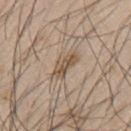Clinical impression:
Imaged during a routine full-body skin examination; the lesion was not biopsied and no histopathology is available.
Image and clinical context:
Imaged with white-light lighting. An algorithmic analysis of the crop reported a footprint of about 5 mm² and an outline eccentricity of about 0.9 (0 = round, 1 = elongated). It also reported a border-irregularity rating of about 4/10, a within-lesion color-variation index near 2/10, and peripheral color asymmetry of about 0.5. Located on the back. The recorded lesion diameter is about 3.5 mm. A 15 mm close-up tile from a total-body photography series done for melanoma screening. The patient is a male roughly 45 years of age.